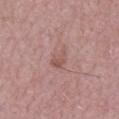Imaged during a routine full-body skin examination; the lesion was not biopsied and no histopathology is available. A 15 mm close-up extracted from a 3D total-body photography capture. The lesion is on the mid back. Captured under white-light illumination. About 3 mm across. A male subject, aged approximately 65. Automated image analysis of the tile measured a shape eccentricity near 0.85 and two-axis asymmetry of about 0.35. The analysis additionally found a lesion color around L≈54 a*≈21 b*≈23 in CIELAB, roughly 8 lightness units darker than nearby skin, and a lesion-to-skin contrast of about 6 (normalized; higher = more distinct).